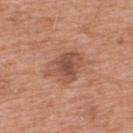This lesion was catalogued during total-body skin photography and was not selected for biopsy.
The patient is a male aged around 70.
The lesion is located on the back.
This is a white-light tile.
An algorithmic analysis of the crop reported a lesion area of about 11 mm² and a shape-asymmetry score of about 0.35 (0 = symmetric).
A 15 mm close-up extracted from a 3D total-body photography capture.
Approximately 5 mm at its widest.